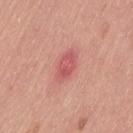• notes — catalogued during a skin exam; not biopsied
• image-analysis metrics — a border-irregularity index near 1.5/10, a color-variation rating of about 3/10, and peripheral color asymmetry of about 1; an automated nevus-likeness rating near 0 out of 100 and a lesion-detection confidence of about 100/100
• imaging modality — total-body-photography crop, ~15 mm field of view
• patient — male, roughly 40 years of age
• site — the left thigh
• illumination — white-light